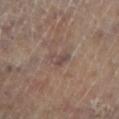{
  "site": "leg",
  "automated_metrics": {
    "area_mm2_approx": 3.0,
    "eccentricity": 0.8,
    "shape_asymmetry": 0.4,
    "cielab_L": 45,
    "cielab_a": 16,
    "cielab_b": 19,
    "vs_skin_darker_L": 7.0,
    "vs_skin_contrast_norm": 6.5,
    "border_irregularity_0_10": 4.0,
    "peripheral_color_asymmetry": 0.5,
    "nevus_likeness_0_100": 0,
    "lesion_detection_confidence_0_100": 85
  },
  "lesion_size": {
    "long_diameter_mm_approx": 2.5
  },
  "patient": {
    "sex": "female",
    "age_approx": 60
  },
  "lighting": "cross-polarized",
  "image": {
    "source": "total-body photography crop",
    "field_of_view_mm": 15
  }
}